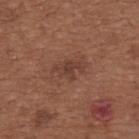notes: no biopsy performed (imaged during a skin exam)
imaging modality: ~15 mm crop, total-body skin-cancer survey
subject: female, approximately 65 years of age
lesion diameter: ~4 mm (longest diameter)
body site: the upper back
tile lighting: white-light illumination